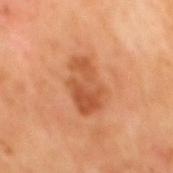{
  "biopsy_status": "not biopsied; imaged during a skin examination",
  "patient": {
    "sex": "male",
    "age_approx": 65
  },
  "lighting": "cross-polarized",
  "site": "mid back",
  "image": {
    "source": "total-body photography crop",
    "field_of_view_mm": 15
  },
  "automated_metrics": {
    "area_mm2_approx": 14.0,
    "eccentricity": 0.8,
    "shape_asymmetry": 0.25,
    "nevus_likeness_0_100": 5,
    "lesion_detection_confidence_0_100": 100
  }
}